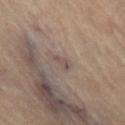Imaged during a routine full-body skin examination; the lesion was not biopsied and no histopathology is available. A 15 mm close-up extracted from a 3D total-body photography capture. Captured under cross-polarized illumination. A female patient, in their mid-60s. About 2.5 mm across. Located on the right lower leg.